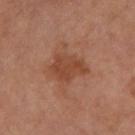Automated tile analysis of the lesion measured a mean CIELAB color near L≈43 a*≈23 b*≈31, about 8 CIELAB-L* units darker than the surrounding skin, and a normalized border contrast of about 7. The analysis additionally found peripheral color asymmetry of about 0.5. It also reported a nevus-likeness score of about 0/100 and lesion-presence confidence of about 100/100.
The subject is a female aged approximately 65.
The tile uses cross-polarized illumination.
Cropped from a whole-body photographic skin survey; the tile spans about 15 mm.
About 4.5 mm across.
On the leg.